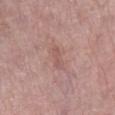{"biopsy_status": "not biopsied; imaged during a skin examination", "patient": {"sex": "female", "age_approx": 60}, "site": "right lower leg", "image": {"source": "total-body photography crop", "field_of_view_mm": 15}}